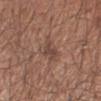Captured during whole-body skin photography for melanoma surveillance; the lesion was not biopsied. Automated tile analysis of the lesion measured a footprint of about 4.5 mm², an outline eccentricity of about 0.65 (0 = round, 1 = elongated), and two-axis asymmetry of about 0.4. And it measured an average lesion color of about L≈44 a*≈18 b*≈25 (CIELAB), roughly 8 lightness units darker than nearby skin, and a lesion-to-skin contrast of about 6.5 (normalized; higher = more distinct). The software also gave a border-irregularity rating of about 4.5/10, a color-variation rating of about 1.5/10, and a peripheral color-asymmetry measure near 0.5. Cropped from a whole-body photographic skin survey; the tile spans about 15 mm. Imaged with white-light lighting. The lesion's longest dimension is about 3 mm. From the arm. The patient is a male aged approximately 40.